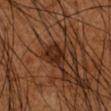notes — total-body-photography surveillance lesion; no biopsy | patient — male, aged approximately 65 | site — the arm | tile lighting — cross-polarized | lesion diameter — ~3.5 mm (longest diameter) | image — 15 mm crop, total-body photography.